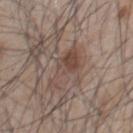biopsy status: no biopsy performed (imaged during a skin exam).